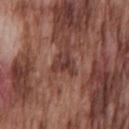notes: catalogued during a skin exam; not biopsied
anatomic site: the chest
lesion diameter: ~2.5 mm (longest diameter)
image source: total-body-photography crop, ~15 mm field of view
TBP lesion metrics: an area of roughly 3.5 mm², an eccentricity of roughly 0.6, and two-axis asymmetry of about 0.55; an average lesion color of about L≈37 a*≈23 b*≈22 (CIELAB), roughly 9 lightness units darker than nearby skin, and a lesion-to-skin contrast of about 8.5 (normalized; higher = more distinct); a border-irregularity index near 6/10 and peripheral color asymmetry of about 0
subject: male, aged around 75
tile lighting: white-light